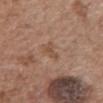Clinical impression:
Recorded during total-body skin imaging; not selected for excision or biopsy.
Acquisition and patient details:
A 15 mm crop from a total-body photograph taken for skin-cancer surveillance. A female patient about 75 years old. On the chest. Measured at roughly 3 mm in maximum diameter. This is a white-light tile.